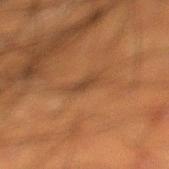Clinical impression:
The lesion was photographed on a routine skin check and not biopsied; there is no pathology result.
Background:
A male subject aged approximately 50. On the left lower leg. A region of skin cropped from a whole-body photographic capture, roughly 15 mm wide. This is a cross-polarized tile. The total-body-photography lesion software estimated a footprint of about 3.5 mm², an eccentricity of roughly 0.85, and two-axis asymmetry of about 0.35. And it measured a mean CIELAB color near L≈34 a*≈17 b*≈28, a lesion–skin lightness drop of about 6, and a normalized lesion–skin contrast near 6. It also reported an automated nevus-likeness rating near 0 out of 100 and a lesion-detection confidence of about 75/100. About 3 mm across.